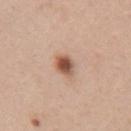lesion_size:
  long_diameter_mm_approx: 2.5
site: chest
image:
  source: total-body photography crop
  field_of_view_mm: 15
patient:
  sex: female
  age_approx: 30